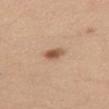<record>
<patient>
  <sex>female</sex>
  <age_approx>35</age_approx>
</patient>
<lighting>white-light</lighting>
<site>abdomen</site>
<image>
  <source>total-body photography crop</source>
  <field_of_view_mm>15</field_of_view_mm>
</image>
</record>